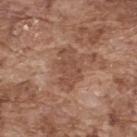Part of a total-body skin-imaging series; this lesion was reviewed on a skin check and was not flagged for biopsy.
On the upper back.
Automated image analysis of the tile measured a lesion area of about 8.5 mm² and a shape eccentricity near 0.85.
A 15 mm close-up extracted from a 3D total-body photography capture.
A male subject aged 73–77.
Longest diameter approximately 4.5 mm.